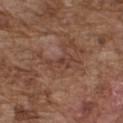<case>
  <biopsy_status>not biopsied; imaged during a skin examination</biopsy_status>
  <patient>
    <sex>male</sex>
    <age_approx>75</age_approx>
  </patient>
  <site>chest</site>
  <image>
    <source>total-body photography crop</source>
    <field_of_view_mm>15</field_of_view_mm>
  </image>
</case>